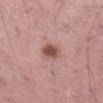notes — catalogued during a skin exam; not biopsied
tile lighting — white-light illumination
size — about 3 mm
image — total-body-photography crop, ~15 mm field of view
patient — male, aged 63 to 67
anatomic site — the left thigh